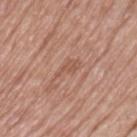| field | value |
|---|---|
| follow-up | catalogued during a skin exam; not biopsied |
| image source | total-body-photography crop, ~15 mm field of view |
| subject | female, aged approximately 80 |
| location | the left thigh |
| lesion size | ~3 mm (longest diameter) |
| automated metrics | a lesion color around L≈54 a*≈22 b*≈29 in CIELAB, a lesion–skin lightness drop of about 7, and a normalized lesion–skin contrast near 5 |
| illumination | white-light illumination |